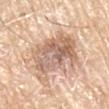<lesion>
  <biopsy_status>not biopsied; imaged during a skin examination</biopsy_status>
  <patient>
    <sex>male</sex>
    <age_approx>80</age_approx>
  </patient>
  <site>left arm</site>
  <lighting>white-light</lighting>
  <image>
    <source>total-body photography crop</source>
    <field_of_view_mm>15</field_of_view_mm>
  </image>
</lesion>